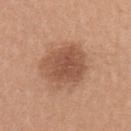Q: Was a biopsy performed?
A: catalogued during a skin exam; not biopsied
Q: Lesion location?
A: the left upper arm
Q: How was this image acquired?
A: 15 mm crop, total-body photography
Q: What are the patient's age and sex?
A: female, roughly 20 years of age
Q: How was the tile lit?
A: white-light